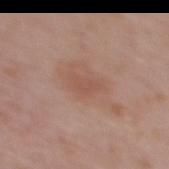Impression: This lesion was catalogued during total-body skin photography and was not selected for biopsy. Acquisition and patient details: Located on the back. Automated image analysis of the tile measured an eccentricity of roughly 0.85 and two-axis asymmetry of about 0.35. The analysis additionally found an average lesion color of about L≈52 a*≈21 b*≈28 (CIELAB) and a normalized border contrast of about 4.5. It also reported lesion-presence confidence of about 100/100. The patient is a female about 60 years old. Imaged with white-light lighting. Cropped from a whole-body photographic skin survey; the tile spans about 15 mm.